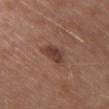Q: Is there a histopathology result?
A: no biopsy performed (imaged during a skin exam)
Q: Where on the body is the lesion?
A: the chest
Q: How was this image acquired?
A: ~15 mm tile from a whole-body skin photo
Q: Automated lesion metrics?
A: a footprint of about 4.5 mm², an outline eccentricity of about 0.8 (0 = round, 1 = elongated), and a symmetry-axis asymmetry near 0.25; a mean CIELAB color near L≈39 a*≈21 b*≈25, about 10 CIELAB-L* units darker than the surrounding skin, and a normalized border contrast of about 8.5
Q: How was the tile lit?
A: white-light
Q: Who is the patient?
A: female, aged approximately 50